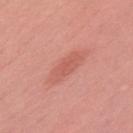Notes:
* workup: no biopsy performed (imaged during a skin exam)
* size: about 5 mm
* anatomic site: the upper back
* image source: ~15 mm crop, total-body skin-cancer survey
* TBP lesion metrics: a lesion–skin lightness drop of about 7 and a normalized border contrast of about 5; a border-irregularity index near 3/10 and a within-lesion color-variation index near 2/10; lesion-presence confidence of about 100/100
* subject: male, in their mid- to late 50s
* illumination: white-light illumination